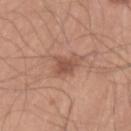workup=catalogued during a skin exam; not biopsied
patient=male, aged 38 to 42
lesion size=~3 mm (longest diameter)
image source=15 mm crop, total-body photography
TBP lesion metrics=a shape eccentricity near 0.7 and two-axis asymmetry of about 0.35; a mean CIELAB color near L≈51 a*≈22 b*≈28 and a normalized lesion–skin contrast near 7; border irregularity of about 3.5 on a 0–10 scale
site=the right lower leg